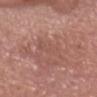Part of a total-body skin-imaging series; this lesion was reviewed on a skin check and was not flagged for biopsy. A male patient approximately 75 years of age. The lesion is located on the head or neck. A 15 mm close-up tile from a total-body photography series done for melanoma screening.